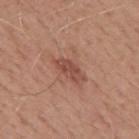Captured during whole-body skin photography for melanoma surveillance; the lesion was not biopsied. A male subject roughly 50 years of age. A region of skin cropped from a whole-body photographic capture, roughly 15 mm wide. On the mid back.